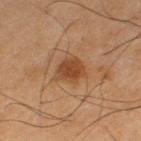The lesion was tiled from a total-body skin photograph and was not biopsied. A male subject in their mid-60s. The recorded lesion diameter is about 3.5 mm. This is a cross-polarized tile. A region of skin cropped from a whole-body photographic capture, roughly 15 mm wide. The lesion is located on the leg.